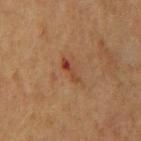Q: Was this lesion biopsied?
A: catalogued during a skin exam; not biopsied
Q: What kind of image is this?
A: total-body-photography crop, ~15 mm field of view
Q: What lighting was used for the tile?
A: cross-polarized
Q: Where on the body is the lesion?
A: the mid back
Q: What are the patient's age and sex?
A: male, about 60 years old
Q: Automated lesion metrics?
A: a mean CIELAB color near L≈38 a*≈24 b*≈30 and a normalized border contrast of about 7.5; a nevus-likeness score of about 0/100 and lesion-presence confidence of about 100/100